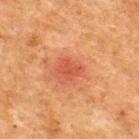| key | value |
|---|---|
| workup | imaged on a skin check; not biopsied |
| lighting | cross-polarized |
| image source | 15 mm crop, total-body photography |
| automated lesion analysis | about 7 CIELAB-L* units darker than the surrounding skin |
| subject | male, about 50 years old |
| body site | the upper back |